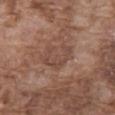biopsy_status: not biopsied; imaged during a skin examination
site: front of the torso
image:
  source: total-body photography crop
  field_of_view_mm: 15
patient:
  sex: male
  age_approx: 75
lesion_size:
  long_diameter_mm_approx: 4.0